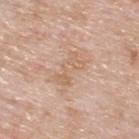Impression:
Captured during whole-body skin photography for melanoma surveillance; the lesion was not biopsied.
Acquisition and patient details:
A roughly 15 mm field-of-view crop from a total-body skin photograph. A male subject aged approximately 60. This is a white-light tile. Automated image analysis of the tile measured border irregularity of about 6.5 on a 0–10 scale, internal color variation of about 2 on a 0–10 scale, and radial color variation of about 1. The software also gave a nevus-likeness score of about 0/100 and a lesion-detection confidence of about 100/100. On the upper back. The lesion's longest dimension is about 4.5 mm.